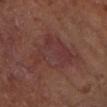Impression:
The lesion was tiled from a total-body skin photograph and was not biopsied.
Context:
Located on the left forearm. The lesion-visualizer software estimated an area of roughly 19 mm², an eccentricity of roughly 0.7, and two-axis asymmetry of about 0.45. The software also gave a border-irregularity index near 6.5/10 and internal color variation of about 4.5 on a 0–10 scale. The analysis additionally found a classifier nevus-likeness of about 0/100. A female subject aged approximately 70. A 15 mm crop from a total-body photograph taken for skin-cancer surveillance.